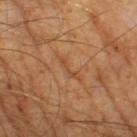Case summary:
* workup · no biopsy performed (imaged during a skin exam)
* size · ~3 mm (longest diameter)
* image source · ~15 mm crop, total-body skin-cancer survey
* illumination · cross-polarized illumination
* subject · male, aged 63 to 67
* automated metrics · roughly 6 lightness units darker than nearby skin and a normalized border contrast of about 5; a classifier nevus-likeness of about 0/100 and a lesion-detection confidence of about 65/100